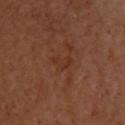Findings:
• anatomic site — the back
• image source — 15 mm crop, total-body photography
• patient — male, aged 63–67
• lesion diameter — about 3 mm